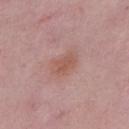The lesion was photographed on a routine skin check and not biopsied; there is no pathology result.
Captured under white-light illumination.
The lesion is located on the chest.
The lesion-visualizer software estimated a shape eccentricity near 0.85 and a shape-asymmetry score of about 0.25 (0 = symmetric).
A close-up tile cropped from a whole-body skin photograph, about 15 mm across.
The recorded lesion diameter is about 4 mm.
A male subject, about 50 years old.